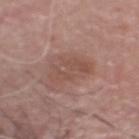| feature | finding |
|---|---|
| notes | total-body-photography surveillance lesion; no biopsy |
| anatomic site | the head or neck |
| tile lighting | white-light illumination |
| acquisition | ~15 mm tile from a whole-body skin photo |
| patient | male, aged 48 to 52 |
| TBP lesion metrics | a mean CIELAB color near L≈50 a*≈20 b*≈24, about 7 CIELAB-L* units darker than the surrounding skin, and a normalized lesion–skin contrast near 5 |
| lesion size | ≈4.5 mm |